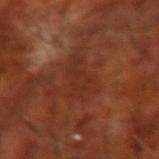Clinical impression:
Imaged during a routine full-body skin examination; the lesion was not biopsied and no histopathology is available.
Acquisition and patient details:
Automated image analysis of the tile measured an area of roughly 9 mm², an eccentricity of roughly 0.85, and a shape-asymmetry score of about 0.4 (0 = symmetric). And it measured a classifier nevus-likeness of about 5/100 and a lesion-detection confidence of about 90/100. A 15 mm close-up tile from a total-body photography series done for melanoma screening. The lesion's longest dimension is about 5 mm. A male patient, aged 68–72. Located on the right forearm. This is a cross-polarized tile.